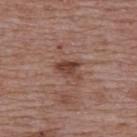Clinical impression: Imaged during a routine full-body skin examination; the lesion was not biopsied and no histopathology is available. Context: The patient is a female approximately 65 years of age. Imaged with white-light lighting. The lesion is located on the upper back. The recorded lesion diameter is about 3 mm. A 15 mm close-up tile from a total-body photography series done for melanoma screening. The total-body-photography lesion software estimated a lesion area of about 4 mm² and a symmetry-axis asymmetry near 0.4.